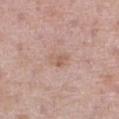Case summary:
- notes: no biopsy performed (imaged during a skin exam)
- lesion size: ~2.5 mm (longest diameter)
- acquisition: ~15 mm crop, total-body skin-cancer survey
- body site: the front of the torso
- subject: male, aged approximately 80
- lighting: white-light